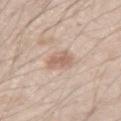This lesion was catalogued during total-body skin photography and was not selected for biopsy.
A male patient aged 43–47.
Imaged with white-light lighting.
The total-body-photography lesion software estimated a border-irregularity rating of about 2/10, internal color variation of about 2 on a 0–10 scale, and a peripheral color-asymmetry measure near 0.5.
From the right upper arm.
Approximately 3 mm at its widest.
A 15 mm crop from a total-body photograph taken for skin-cancer surveillance.